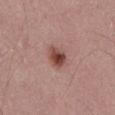Imaged during a routine full-body skin examination; the lesion was not biopsied and no histopathology is available. The patient is a male about 55 years old. A 15 mm crop from a total-body photograph taken for skin-cancer surveillance. An algorithmic analysis of the crop reported a normalized border contrast of about 9.5. The lesion's longest dimension is about 3 mm. Imaged with white-light lighting. The lesion is on the abdomen.